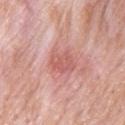biopsy status: no biopsy performed (imaged during a skin exam)
anatomic site: the back
size: ~3.5 mm (longest diameter)
image: ~15 mm crop, total-body skin-cancer survey
image-analysis metrics: a lesion area of about 7 mm², an outline eccentricity of about 0.65 (0 = round, 1 = elongated), and two-axis asymmetry of about 0.2; an average lesion color of about L≈59 a*≈28 b*≈26 (CIELAB), roughly 9 lightness units darker than nearby skin, and a lesion-to-skin contrast of about 6 (normalized; higher = more distinct)
tile lighting: white-light
patient: male, roughly 55 years of age